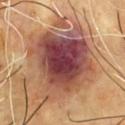Case summary:
– workup: catalogued during a skin exam; not biopsied
– subject: male, about 65 years old
– anatomic site: the chest
– lesion diameter: ≈8.5 mm
– acquisition: ~15 mm crop, total-body skin-cancer survey
– lighting: cross-polarized